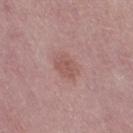| field | value |
|---|---|
| biopsy status | imaged on a skin check; not biopsied |
| TBP lesion metrics | a border-irregularity rating of about 2/10 and peripheral color asymmetry of about 0.5; a nevus-likeness score of about 5/100 |
| lighting | white-light |
| diameter | ~3 mm (longest diameter) |
| patient | female, aged approximately 50 |
| location | the right thigh |
| acquisition | 15 mm crop, total-body photography |